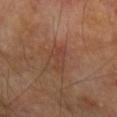No biopsy was performed on this lesion — it was imaged during a full skin examination and was not determined to be concerning.